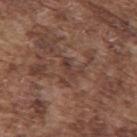biopsy status: no biopsy performed (imaged during a skin exam) | tile lighting: white-light illumination | image: ~15 mm tile from a whole-body skin photo | subject: male, roughly 75 years of age | site: the upper back | lesion size: ≈2.5 mm | automated metrics: an average lesion color of about L≈38 a*≈19 b*≈23 (CIELAB), about 7 CIELAB-L* units darker than the surrounding skin, and a lesion-to-skin contrast of about 6.5 (normalized; higher = more distinct); a nevus-likeness score of about 0/100 and lesion-presence confidence of about 55/100.